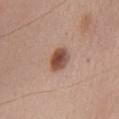A close-up tile cropped from a whole-body skin photograph, about 15 mm across.
A male patient roughly 65 years of age.
On the chest.
The tile uses white-light illumination.
Automated tile analysis of the lesion measured an area of roughly 6 mm² and a symmetry-axis asymmetry near 0.2. It also reported about 15 CIELAB-L* units darker than the surrounding skin and a lesion-to-skin contrast of about 10.5 (normalized; higher = more distinct).